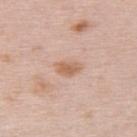Assessment:
No biopsy was performed on this lesion — it was imaged during a full skin examination and was not determined to be concerning.
Image and clinical context:
This is a white-light tile. The lesion is on the upper back. A female subject, aged approximately 65. The total-body-photography lesion software estimated a shape eccentricity near 0.8 and two-axis asymmetry of about 0.25. The software also gave a normalized border contrast of about 7.5. The software also gave a border-irregularity rating of about 2.5/10. A 15 mm close-up extracted from a 3D total-body photography capture.